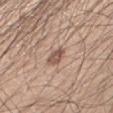Q: Was this lesion biopsied?
A: catalogued during a skin exam; not biopsied
Q: What is the lesion's diameter?
A: ≈2.5 mm
Q: Automated lesion metrics?
A: a lesion color around L≈53 a*≈19 b*≈25 in CIELAB and roughly 12 lightness units darker than nearby skin; a border-irregularity rating of about 2/10, a within-lesion color-variation index near 2/10, and radial color variation of about 1; a lesion-detection confidence of about 100/100
Q: What is the imaging modality?
A: 15 mm crop, total-body photography
Q: What is the anatomic site?
A: the arm
Q: Who is the patient?
A: male, in their 30s
Q: How was the tile lit?
A: white-light illumination